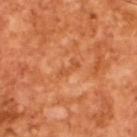Case summary:
• notes: imaged on a skin check; not biopsied
• imaging modality: ~15 mm crop, total-body skin-cancer survey
• lesion size: about 3 mm
• tile lighting: cross-polarized
• patient: male, about 65 years old